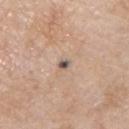Acquisition and patient details:
A male subject approximately 60 years of age. An algorithmic analysis of the crop reported an automated nevus-likeness rating near 10 out of 100 and a detector confidence of about 100 out of 100 that the crop contains a lesion. Imaged with white-light lighting. The recorded lesion diameter is about 1.5 mm. A 15 mm close-up extracted from a 3D total-body photography capture. From the chest.
Conclusion:
On excision, pathology confirmed a squamous cell carcinoma in situ.